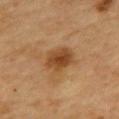Impression:
Captured during whole-body skin photography for melanoma surveillance; the lesion was not biopsied.
Clinical summary:
From the upper back. A female subject aged 58 to 62. This image is a 15 mm lesion crop taken from a total-body photograph.